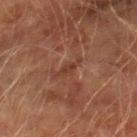Findings:
• biopsy status — catalogued during a skin exam; not biopsied